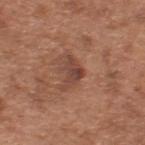Q: Is there a histopathology result?
A: imaged on a skin check; not biopsied